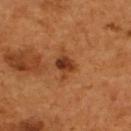{
  "biopsy_status": "not biopsied; imaged during a skin examination",
  "lighting": "cross-polarized",
  "patient": {
    "sex": "male",
    "age_approx": 55
  },
  "lesion_size": {
    "long_diameter_mm_approx": 2.5
  },
  "site": "back",
  "automated_metrics": {
    "eccentricity": 0.55,
    "shape_asymmetry": 0.35,
    "cielab_L": 35,
    "cielab_a": 24,
    "cielab_b": 33,
    "vs_skin_darker_L": 11.0,
    "vs_skin_contrast_norm": 9.5
  },
  "image": {
    "source": "total-body photography crop",
    "field_of_view_mm": 15
  }
}